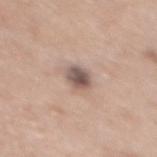biopsy status — catalogued during a skin exam; not biopsied | image — 15 mm crop, total-body photography | patient — female, aged 58–62 | location — the upper back.